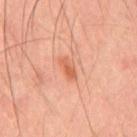body site: the mid back | subject: male, in their mid- to late 40s | image source: total-body-photography crop, ~15 mm field of view | illumination: cross-polarized.